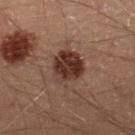automated metrics = a footprint of about 12 mm², a shape eccentricity near 0.2, and two-axis asymmetry of about 0.25; a border-irregularity rating of about 2.5/10, internal color variation of about 5.5 on a 0–10 scale, and radial color variation of about 2
site = the right lower leg
lesion diameter = about 4 mm
patient = male, in their 40s
acquisition = 15 mm crop, total-body photography
lighting = cross-polarized